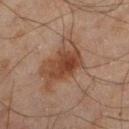• biopsy status · imaged on a skin check; not biopsied
• diameter · ~4 mm (longest diameter)
• imaging modality · ~15 mm tile from a whole-body skin photo
• tile lighting · cross-polarized illumination
• body site · the left lower leg
• subject · male, in their mid-40s